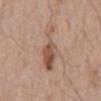Clinical impression:
Captured during whole-body skin photography for melanoma surveillance; the lesion was not biopsied.
Background:
The patient is a male aged 58 to 62. The tile uses white-light illumination. The lesion is on the mid back. Longest diameter approximately 8.5 mm. A lesion tile, about 15 mm wide, cut from a 3D total-body photograph. An algorithmic analysis of the crop reported a footprint of about 21 mm², an outline eccentricity of about 0.9 (0 = round, 1 = elongated), and two-axis asymmetry of about 0.45. It also reported a normalized lesion–skin contrast near 5.5. The analysis additionally found a border-irregularity index near 7.5/10 and a peripheral color-asymmetry measure near 3. And it measured a nevus-likeness score of about 85/100 and a lesion-detection confidence of about 95/100.